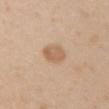Recorded during total-body skin imaging; not selected for excision or biopsy.
A female patient, roughly 30 years of age.
On the arm.
This is a white-light tile.
A lesion tile, about 15 mm wide, cut from a 3D total-body photograph.